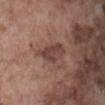No biopsy was performed on this lesion — it was imaged during a full skin examination and was not determined to be concerning.
Longest diameter approximately 4.5 mm.
A lesion tile, about 15 mm wide, cut from a 3D total-body photograph.
The subject is a male approximately 75 years of age.
The lesion is located on the abdomen.
Automated image analysis of the tile measured a shape eccentricity near 0.85 and a shape-asymmetry score of about 0.25 (0 = symmetric). And it measured border irregularity of about 3 on a 0–10 scale, internal color variation of about 3 on a 0–10 scale, and radial color variation of about 1.5. And it measured a classifier nevus-likeness of about 0/100 and a lesion-detection confidence of about 100/100.